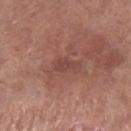notes=no biopsy performed (imaged during a skin exam) | lighting=white-light | imaging modality=~15 mm tile from a whole-body skin photo | diameter=about 3 mm | location=the left lower leg | subject=male, aged 68 to 72.